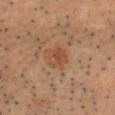{
  "patient": {
    "sex": "male",
    "age_approx": 65
  },
  "image": {
    "source": "total-body photography crop",
    "field_of_view_mm": 15
  },
  "lighting": "cross-polarized",
  "lesion_size": {
    "long_diameter_mm_approx": 2.5
  },
  "site": "chest"
}